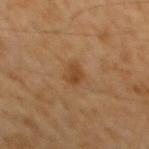workup: total-body-photography surveillance lesion; no biopsy | tile lighting: cross-polarized | body site: the back | patient: male, approximately 60 years of age | automated lesion analysis: a footprint of about 3.5 mm² and a shape eccentricity near 0.75; an automated nevus-likeness rating near 70 out of 100 and a detector confidence of about 100 out of 100 that the crop contains a lesion | image source: ~15 mm tile from a whole-body skin photo.